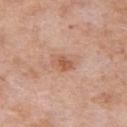<lesion>
<biopsy_status>not biopsied; imaged during a skin examination</biopsy_status>
<image>
  <source>total-body photography crop</source>
  <field_of_view_mm>15</field_of_view_mm>
</image>
<site>arm</site>
<patient>
  <sex>female</sex>
  <age_approx>70</age_approx>
</patient>
</lesion>